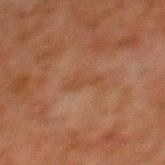Impression: Part of a total-body skin-imaging series; this lesion was reviewed on a skin check and was not flagged for biopsy. Background: A male subject aged around 80. The recorded lesion diameter is about 3 mm. A close-up tile cropped from a whole-body skin photograph, about 15 mm across. Located on the back. Imaged with cross-polarized lighting.